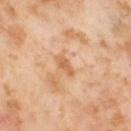biopsy status: total-body-photography surveillance lesion; no biopsy | patient: female, aged 53–57 | site: the left thigh | tile lighting: cross-polarized illumination | imaging modality: ~15 mm crop, total-body skin-cancer survey.